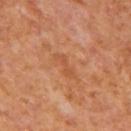  biopsy_status: not biopsied; imaged during a skin examination
  lighting: cross-polarized
  lesion_size:
    long_diameter_mm_approx: 3.5
  patient:
    sex: male
    age_approx: 65
  image:
    source: total-body photography crop
    field_of_view_mm: 15
  site: back